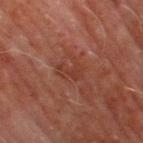imaging modality = ~15 mm tile from a whole-body skin photo; illumination = cross-polarized illumination; lesion diameter = ≈3 mm; location = the leg; patient = male, in their 70s.